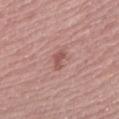Assessment: This lesion was catalogued during total-body skin photography and was not selected for biopsy. Background: This image is a 15 mm lesion crop taken from a total-body photograph. On the right forearm. Automated tile analysis of the lesion measured an area of roughly 3 mm², an outline eccentricity of about 0.8 (0 = round, 1 = elongated), and a shape-asymmetry score of about 0.35 (0 = symmetric). The software also gave an average lesion color of about L≈53 a*≈24 b*≈23 (CIELAB), roughly 9 lightness units darker than nearby skin, and a normalized border contrast of about 6.5. The analysis additionally found a within-lesion color-variation index near 1/10 and a peripheral color-asymmetry measure near 0.5. It also reported a nevus-likeness score of about 0/100 and a detector confidence of about 100 out of 100 that the crop contains a lesion. The tile uses white-light illumination. A female patient in their mid-60s.